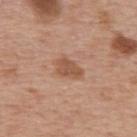Acquisition and patient details: A 15 mm close-up tile from a total-body photography series done for melanoma screening. The recorded lesion diameter is about 3.5 mm. Imaged with white-light lighting. The lesion-visualizer software estimated a lesion area of about 5.5 mm², an outline eccentricity of about 0.85 (0 = round, 1 = elongated), and a shape-asymmetry score of about 0.25 (0 = symmetric). And it measured a mean CIELAB color near L≈53 a*≈22 b*≈31, a lesion–skin lightness drop of about 10, and a normalized lesion–skin contrast near 7. The software also gave a nevus-likeness score of about 50/100 and a detector confidence of about 100 out of 100 that the crop contains a lesion. A male subject, aged 73–77. From the upper back.